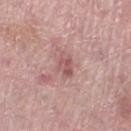Part of a total-body skin-imaging series; this lesion was reviewed on a skin check and was not flagged for biopsy.
From the right lower leg.
The subject is a female aged 58 to 62.
This image is a 15 mm lesion crop taken from a total-body photograph.